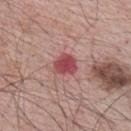Assessment: No biopsy was performed on this lesion — it was imaged during a full skin examination and was not determined to be concerning. Background: The patient is a male in their mid- to late 60s. A lesion tile, about 15 mm wide, cut from a 3D total-body photograph. Longest diameter approximately 3 mm. An algorithmic analysis of the crop reported a footprint of about 6 mm² and a shape-asymmetry score of about 0.25 (0 = symmetric). And it measured a lesion color around L≈48 a*≈31 b*≈22 in CIELAB and a normalized lesion–skin contrast near 9.5. The analysis additionally found a classifier nevus-likeness of about 10/100 and lesion-presence confidence of about 100/100. From the upper back. This is a white-light tile.